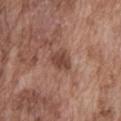Notes:
* biopsy status · catalogued during a skin exam; not biopsied
* illumination · white-light illumination
* subject · male, aged around 75
* imaging modality · ~15 mm tile from a whole-body skin photo
* lesion size · ≈3 mm
* site · the front of the torso
* TBP lesion metrics · about 10 CIELAB-L* units darker than the surrounding skin and a lesion-to-skin contrast of about 8 (normalized; higher = more distinct); a nevus-likeness score of about 10/100 and a lesion-detection confidence of about 100/100